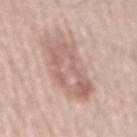| key | value |
|---|---|
| image-analysis metrics | a footprint of about 21 mm², a shape eccentricity near 0.85, and a symmetry-axis asymmetry near 0.2; roughly 10 lightness units darker than nearby skin; a border-irregularity rating of about 4/10 and radial color variation of about 2; a classifier nevus-likeness of about 5/100 |
| patient | male, aged 58 to 62 |
| illumination | white-light illumination |
| size | about 7 mm |
| acquisition | total-body-photography crop, ~15 mm field of view |
| anatomic site | the mid back |